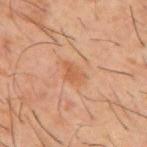follow-up: imaged on a skin check; not biopsied
size: ~3 mm (longest diameter)
acquisition: ~15 mm crop, total-body skin-cancer survey
anatomic site: the mid back
subject: male, aged around 60
illumination: cross-polarized